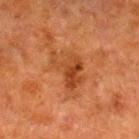Acquisition and patient details: A male subject approximately 80 years of age. The lesion is on the right lower leg. Cropped from a whole-body photographic skin survey; the tile spans about 15 mm.